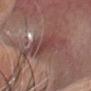  biopsy_status: not biopsied; imaged during a skin examination
  site: head or neck
  lighting: white-light
  patient:
    sex: male
    age_approx: 65
  lesion_size:
    long_diameter_mm_approx: 6.5
  image:
    source: total-body photography crop
    field_of_view_mm: 15
  automated_metrics:
    area_mm2_approx: 18.0
    eccentricity: 0.85
    shape_asymmetry: 0.25
    nevus_likeness_0_100: 0